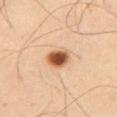follow-up: catalogued during a skin exam; not biopsied | lesion size: ~3 mm (longest diameter) | patient: male, in their mid-50s | illumination: cross-polarized | image: ~15 mm tile from a whole-body skin photo | location: the abdomen.